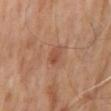No biopsy was performed on this lesion — it was imaged during a full skin examination and was not determined to be concerning. A male subject aged approximately 65. The total-body-photography lesion software estimated a footprint of about 3.5 mm², a shape eccentricity near 0.65, and a shape-asymmetry score of about 0.15 (0 = symmetric). The analysis additionally found a mean CIELAB color near L≈47 a*≈22 b*≈30 and a normalized lesion–skin contrast near 5.5. Located on the mid back. Captured under cross-polarized illumination. Cropped from a whole-body photographic skin survey; the tile spans about 15 mm. Measured at roughly 2.5 mm in maximum diameter.